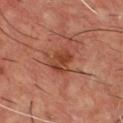Clinical impression: Part of a total-body skin-imaging series; this lesion was reviewed on a skin check and was not flagged for biopsy. Context: The lesion is on the front of the torso. A region of skin cropped from a whole-body photographic capture, roughly 15 mm wide. Captured under cross-polarized illumination. The lesion's longest dimension is about 3 mm. A male patient, in their mid- to late 50s. The total-body-photography lesion software estimated a footprint of about 6.5 mm² and an outline eccentricity of about 0.55 (0 = round, 1 = elongated). And it measured a mean CIELAB color near L≈41 a*≈27 b*≈31, a lesion–skin lightness drop of about 9, and a lesion-to-skin contrast of about 7 (normalized; higher = more distinct). It also reported a border-irregularity rating of about 2.5/10, internal color variation of about 4.5 on a 0–10 scale, and a peripheral color-asymmetry measure near 1.5. The analysis additionally found a nevus-likeness score of about 0/100 and a lesion-detection confidence of about 100/100.